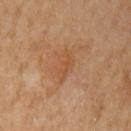biopsy status = total-body-photography surveillance lesion; no biopsy | subject = female, aged 58–62 | imaging modality = total-body-photography crop, ~15 mm field of view | location = the left upper arm.